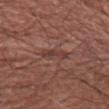Impression: The lesion was tiled from a total-body skin photograph and was not biopsied. Background: This image is a 15 mm lesion crop taken from a total-body photograph. This is a white-light tile. A male patient, about 65 years old. On the right upper arm.